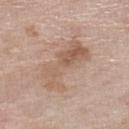No biopsy was performed on this lesion — it was imaged during a full skin examination and was not determined to be concerning. The lesion is on the leg. An algorithmic analysis of the crop reported an area of roughly 17 mm², an outline eccentricity of about 0.9 (0 = round, 1 = elongated), and a symmetry-axis asymmetry near 0.35. The analysis additionally found a nevus-likeness score of about 0/100. This is a white-light tile. A 15 mm crop from a total-body photograph taken for skin-cancer surveillance. A female subject aged 73–77. Approximately 7 mm at its widest.